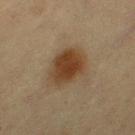Q: Was a biopsy performed?
A: total-body-photography surveillance lesion; no biopsy
Q: What is the imaging modality?
A: ~15 mm tile from a whole-body skin photo
Q: What is the anatomic site?
A: the right thigh
Q: What are the patient's age and sex?
A: female, aged 53–57